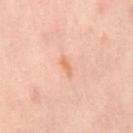Acquisition and patient details: From the left leg. A 15 mm close-up extracted from a 3D total-body photography capture. The subject is a female aged around 40.